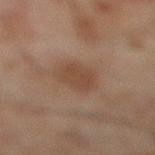  automated_metrics:
    nevus_likeness_0_100: 45
  patient:
    sex: male
    age_approx: 45
  image:
    source: total-body photography crop
    field_of_view_mm: 15
  site: leg
  lesion_size:
    long_diameter_mm_approx: 3.5